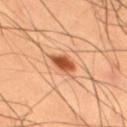No biopsy was performed on this lesion — it was imaged during a full skin examination and was not determined to be concerning.
The patient is a male about 50 years old.
Cropped from a total-body skin-imaging series; the visible field is about 15 mm.
The recorded lesion diameter is about 2.5 mm.
On the right thigh.